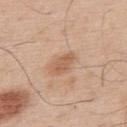<lesion>
<biopsy_status>not biopsied; imaged during a skin examination</biopsy_status>
<lesion_size>
  <long_diameter_mm_approx>3.0</long_diameter_mm_approx>
</lesion_size>
<site>upper back</site>
<lighting>white-light</lighting>
<patient>
  <sex>male</sex>
  <age_approx>55</age_approx>
</patient>
<automated_metrics>
  <nevus_likeness_0_100>85</nevus_likeness_0_100>
  <lesion_detection_confidence_0_100>100</lesion_detection_confidence_0_100>
</automated_metrics>
<image>
  <source>total-body photography crop</source>
  <field_of_view_mm>15</field_of_view_mm>
</image>
</lesion>